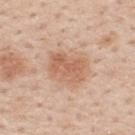Impression:
No biopsy was performed on this lesion — it was imaged during a full skin examination and was not determined to be concerning.
Clinical summary:
The lesion-visualizer software estimated a lesion area of about 11 mm², an eccentricity of roughly 0.7, and a symmetry-axis asymmetry near 0.2. It also reported an average lesion color of about L≈61 a*≈22 b*≈32 (CIELAB) and a normalized border contrast of about 6.5. The software also gave a border-irregularity rating of about 2.5/10, a within-lesion color-variation index near 3.5/10, and radial color variation of about 1.5. The analysis additionally found lesion-presence confidence of about 100/100. Longest diameter approximately 4 mm. Captured under white-light illumination. The subject is a male aged 58–62. A 15 mm close-up tile from a total-body photography series done for melanoma screening. The lesion is located on the mid back.